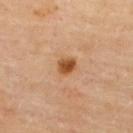Captured during whole-body skin photography for melanoma surveillance; the lesion was not biopsied.
A female patient about 60 years old.
The total-body-photography lesion software estimated a shape eccentricity near 0.5 and two-axis asymmetry of about 0.25. It also reported an automated nevus-likeness rating near 95 out of 100.
A lesion tile, about 15 mm wide, cut from a 3D total-body photograph.
Imaged with cross-polarized lighting.
Located on the upper back.